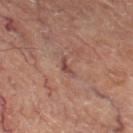biopsy_status: not biopsied; imaged during a skin examination
automated_metrics:
  area_mm2_approx: 2.0
  eccentricity: 0.9
  shape_asymmetry: 0.55
  cielab_L: 45
  cielab_a: 21
  cielab_b: 23
  vs_skin_contrast_norm: 7.0
  border_irregularity_0_10: 5.5
  peripheral_color_asymmetry: 0.0
  nevus_likeness_0_100: 0
site: left thigh
lesion_size:
  long_diameter_mm_approx: 2.5
lighting: cross-polarized
patient:
  sex: male
  age_approx: 70
image:
  source: total-body photography crop
  field_of_view_mm: 15